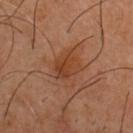| key | value |
|---|---|
| notes | imaged on a skin check; not biopsied |
| image | ~15 mm tile from a whole-body skin photo |
| site | the chest |
| patient | male, aged around 50 |